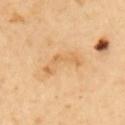Q: Was a biopsy performed?
A: no biopsy performed (imaged during a skin exam)
Q: Who is the patient?
A: male, aged around 65
Q: What kind of image is this?
A: total-body-photography crop, ~15 mm field of view
Q: What is the anatomic site?
A: the upper back
Q: What did automated image analysis measure?
A: a shape eccentricity near 0.95 and two-axis asymmetry of about 0.55; border irregularity of about 6.5 on a 0–10 scale and peripheral color asymmetry of about 1
Q: How large is the lesion?
A: ~6 mm (longest diameter)
Q: What lighting was used for the tile?
A: cross-polarized illumination